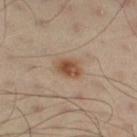  biopsy_status: not biopsied; imaged during a skin examination
  lesion_size:
    long_diameter_mm_approx: 3.0
  automated_metrics:
    area_mm2_approx: 6.5
    eccentricity: 0.65
    shape_asymmetry: 0.25
    cielab_L: 51
    cielab_a: 18
    cielab_b: 31
    nevus_likeness_0_100: 95
    lesion_detection_confidence_0_100: 100
  site: leg
  patient:
    sex: male
    age_approx: 55
  lighting: cross-polarized
  image:
    source: total-body photography crop
    field_of_view_mm: 15